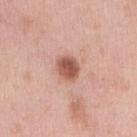Assessment:
Part of a total-body skin-imaging series; this lesion was reviewed on a skin check and was not flagged for biopsy.
Acquisition and patient details:
This is a white-light tile. An algorithmic analysis of the crop reported an area of roughly 6 mm², an eccentricity of roughly 0.45, and two-axis asymmetry of about 0.25. The software also gave a normalized border contrast of about 9.5. And it measured an automated nevus-likeness rating near 95 out of 100. The lesion's longest dimension is about 2.5 mm. From the right upper arm. Cropped from a total-body skin-imaging series; the visible field is about 15 mm. A female subject aged 48 to 52.